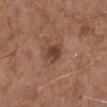This lesion was catalogued during total-body skin photography and was not selected for biopsy.
On the leg.
A lesion tile, about 15 mm wide, cut from a 3D total-body photograph.
Automated image analysis of the tile measured a lesion area of about 5.5 mm², an eccentricity of roughly 0.5, and a shape-asymmetry score of about 0.2 (0 = symmetric). The software also gave an average lesion color of about L≈41 a*≈20 b*≈24 (CIELAB) and a normalized border contrast of about 9. It also reported a border-irregularity rating of about 2/10, a color-variation rating of about 3/10, and radial color variation of about 1.
The patient is a male about 60 years old.